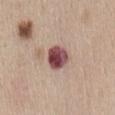workup = no biopsy performed (imaged during a skin exam).